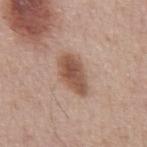Findings:
* site · the abdomen
* subject · male, roughly 65 years of age
* acquisition · total-body-photography crop, ~15 mm field of view
* diameter · ≈5 mm
* lighting · white-light illumination
* automated lesion analysis · a nevus-likeness score of about 90/100 and a lesion-detection confidence of about 100/100